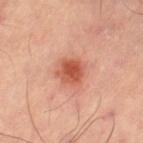The lesion was photographed on a routine skin check and not biopsied; there is no pathology result. A region of skin cropped from a whole-body photographic capture, roughly 15 mm wide. The lesion is on the leg. Captured under cross-polarized illumination. About 3 mm across.